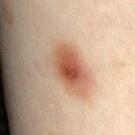Part of a total-body skin-imaging series; this lesion was reviewed on a skin check and was not flagged for biopsy. An algorithmic analysis of the crop reported a lesion area of about 16 mm². The software also gave a border-irregularity index near 2/10, internal color variation of about 8.5 on a 0–10 scale, and radial color variation of about 2. The lesion is located on the back. A female subject approximately 55 years of age. Imaged with cross-polarized lighting. Cropped from a whole-body photographic skin survey; the tile spans about 15 mm. The recorded lesion diameter is about 5.5 mm.